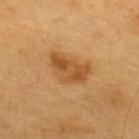Impression:
Part of a total-body skin-imaging series; this lesion was reviewed on a skin check and was not flagged for biopsy.
Context:
Automated image analysis of the tile measured a shape eccentricity near 0.85. And it measured about 10 CIELAB-L* units darker than the surrounding skin and a lesion-to-skin contrast of about 7.5 (normalized; higher = more distinct). This is a cross-polarized tile. This image is a 15 mm lesion crop taken from a total-body photograph. On the back. A male patient aged 58–62. Approximately 5 mm at its widest.